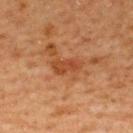workup: imaged on a skin check; not biopsied
subject: male, aged around 65
image source: 15 mm crop, total-body photography
anatomic site: the upper back